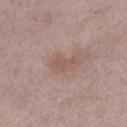{
  "biopsy_status": "not biopsied; imaged during a skin examination",
  "site": "left lower leg",
  "lesion_size": {
    "long_diameter_mm_approx": 3.5
  },
  "patient": {
    "sex": "female",
    "age_approx": 60
  },
  "lighting": "white-light",
  "image": {
    "source": "total-body photography crop",
    "field_of_view_mm": 15
  }
}